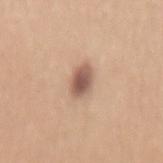Clinical impression:
Captured during whole-body skin photography for melanoma surveillance; the lesion was not biopsied.
Clinical summary:
A male subject approximately 40 years of age. The tile uses white-light illumination. Cropped from a whole-body photographic skin survey; the tile spans about 15 mm. From the mid back. About 3.5 mm across.